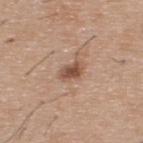follow-up: total-body-photography surveillance lesion; no biopsy
body site: the back
image-analysis metrics: a border-irregularity rating of about 2.5/10, internal color variation of about 4 on a 0–10 scale, and a peripheral color-asymmetry measure near 1
image: total-body-photography crop, ~15 mm field of view
illumination: white-light
patient: male, roughly 40 years of age